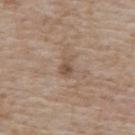Assessment: The lesion was photographed on a routine skin check and not biopsied; there is no pathology result. Clinical summary: Cropped from a total-body skin-imaging series; the visible field is about 15 mm. A female patient aged 73–77. This is a white-light tile. Automated tile analysis of the lesion measured a shape eccentricity near 0.8 and a symmetry-axis asymmetry near 0.35. And it measured an average lesion color of about L≈51 a*≈15 b*≈27 (CIELAB), about 9 CIELAB-L* units darker than the surrounding skin, and a normalized lesion–skin contrast near 6.5. The analysis additionally found lesion-presence confidence of about 100/100. On the upper back.